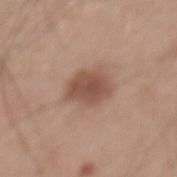workup: no biopsy performed (imaged during a skin exam)
imaging modality: 15 mm crop, total-body photography
patient: male, about 65 years old
size: about 4 mm
site: the mid back